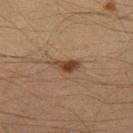Findings:
- workup — total-body-photography surveillance lesion; no biopsy
- anatomic site — the right thigh
- patient — male, approximately 50 years of age
- imaging modality — total-body-photography crop, ~15 mm field of view
- automated lesion analysis — border irregularity of about 3.5 on a 0–10 scale and a within-lesion color-variation index near 5/10; a nevus-likeness score of about 95/100
- illumination — cross-polarized